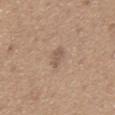Q: Was a biopsy performed?
A: total-body-photography surveillance lesion; no biopsy
Q: What are the patient's age and sex?
A: male, approximately 65 years of age
Q: Illumination type?
A: white-light
Q: What is the anatomic site?
A: the mid back
Q: How was this image acquired?
A: ~15 mm tile from a whole-body skin photo
Q: How large is the lesion?
A: about 2.5 mm